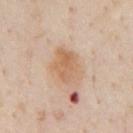  biopsy_status: not biopsied; imaged during a skin examination
  lesion_size:
    long_diameter_mm_approx: 4.0
  patient:
    sex: male
    age_approx: 55
  image:
    source: total-body photography crop
    field_of_view_mm: 15
  lighting: cross-polarized
  automated_metrics:
    vs_skin_darker_L: 9.0
    vs_skin_contrast_norm: 7.0
    border_irregularity_0_10: 2.5
    color_variation_0_10: 4.0
    peripheral_color_asymmetry: 1.5
    nevus_likeness_0_100: 95
    lesion_detection_confidence_0_100: 100
  site: chest